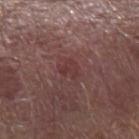Case summary:
• follow-up · imaged on a skin check; not biopsied
• subject · male, aged 73–77
• anatomic site · the arm
• imaging modality · total-body-photography crop, ~15 mm field of view
• lesion diameter · ~2.5 mm (longest diameter)
• illumination · white-light